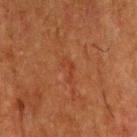Assessment: No biopsy was performed on this lesion — it was imaged during a full skin examination and was not determined to be concerning.